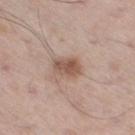No biopsy was performed on this lesion — it was imaged during a full skin examination and was not determined to be concerning. Cropped from a whole-body photographic skin survey; the tile spans about 15 mm. Captured under white-light illumination. A male subject, aged approximately 55. On the right thigh. The total-body-photography lesion software estimated a footprint of about 6.5 mm², an outline eccentricity of about 0.75 (0 = round, 1 = elongated), and two-axis asymmetry of about 0.25. The analysis additionally found an automated nevus-likeness rating near 80 out of 100. Longest diameter approximately 3.5 mm.